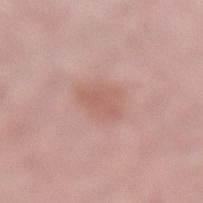The lesion was tiled from a total-body skin photograph and was not biopsied. The lesion is on the left lower leg. A female patient in their mid- to late 60s. Approximately 3.5 mm at its widest. A close-up tile cropped from a whole-body skin photograph, about 15 mm across.